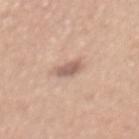Case summary:
• notes — no biopsy performed (imaged during a skin exam)
• illumination — white-light
• lesion diameter — about 2.5 mm
• acquisition — 15 mm crop, total-body photography
• subject — female, aged 28–32
• anatomic site — the back